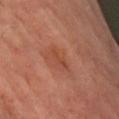Q: Was this lesion biopsied?
A: no biopsy performed (imaged during a skin exam)
Q: What is the anatomic site?
A: the right upper arm
Q: How was this image acquired?
A: total-body-photography crop, ~15 mm field of view
Q: Patient demographics?
A: male, about 70 years old